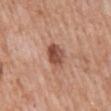The lesion was photographed on a routine skin check and not biopsied; there is no pathology result. A male subject aged around 55. Approximately 3 mm at its widest. The lesion-visualizer software estimated a border-irregularity rating of about 1.5/10, internal color variation of about 5.5 on a 0–10 scale, and a peripheral color-asymmetry measure near 2. Located on the back. A 15 mm close-up extracted from a 3D total-body photography capture.